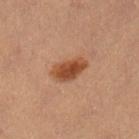Captured during whole-body skin photography for melanoma surveillance; the lesion was not biopsied. The subject is a female aged around 35. The total-body-photography lesion software estimated a footprint of about 8 mm², a shape eccentricity near 0.85, and a symmetry-axis asymmetry near 0.2. And it measured a mean CIELAB color near L≈46 a*≈24 b*≈34, roughly 12 lightness units darker than nearby skin, and a normalized lesion–skin contrast near 9.5. It also reported a nevus-likeness score of about 100/100 and lesion-presence confidence of about 100/100. The recorded lesion diameter is about 4.5 mm. A 15 mm close-up extracted from a 3D total-body photography capture. The lesion is on the leg.